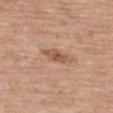<record>
  <biopsy_status>not biopsied; imaged during a skin examination</biopsy_status>
  <site>back</site>
  <image>
    <source>total-body photography crop</source>
    <field_of_view_mm>15</field_of_view_mm>
  </image>
  <lesion_size>
    <long_diameter_mm_approx>5.0</long_diameter_mm_approx>
  </lesion_size>
  <patient>
    <sex>male</sex>
    <age_approx>80</age_approx>
  </patient>
  <lighting>white-light</lighting>
</record>